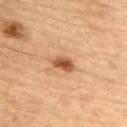Impression:
Captured during whole-body skin photography for melanoma surveillance; the lesion was not biopsied.
Clinical summary:
This image is a 15 mm lesion crop taken from a total-body photograph. A male subject, aged approximately 85 or older. On the upper back.